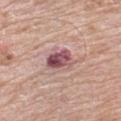The lesion was photographed on a routine skin check and not biopsied; there is no pathology result.
Longest diameter approximately 3.5 mm.
On the left upper arm.
The subject is a male in their mid-50s.
A lesion tile, about 15 mm wide, cut from a 3D total-body photograph.